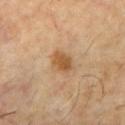Q: Is there a histopathology result?
A: total-body-photography surveillance lesion; no biopsy
Q: Who is the patient?
A: male, in their 60s
Q: How was this image acquired?
A: total-body-photography crop, ~15 mm field of view
Q: What is the anatomic site?
A: the chest
Q: How was the tile lit?
A: cross-polarized illumination